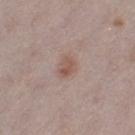Impression: The lesion was tiled from a total-body skin photograph and was not biopsied. Clinical summary: Measured at roughly 2.5 mm in maximum diameter. Located on the left thigh. Imaged with white-light lighting. A region of skin cropped from a whole-body photographic capture, roughly 15 mm wide. The patient is a female aged around 30.